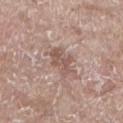follow-up = no biopsy performed (imaged during a skin exam); imaging modality = ~15 mm crop, total-body skin-cancer survey; anatomic site = the leg; subject = female, aged around 85 or older; lighting = white-light.